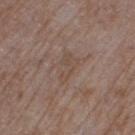The lesion was tiled from a total-body skin photograph and was not biopsied.
A roughly 15 mm field-of-view crop from a total-body skin photograph.
The total-body-photography lesion software estimated a footprint of about 5 mm², an outline eccentricity of about 0.85 (0 = round, 1 = elongated), and two-axis asymmetry of about 0.45.
From the left thigh.
This is a white-light tile.
A male subject in their mid-80s or older.